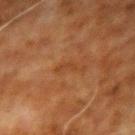Recorded during total-body skin imaging; not selected for excision or biopsy. A roughly 15 mm field-of-view crop from a total-body skin photograph. The lesion is on the right upper arm. A male subject aged 68 to 72.